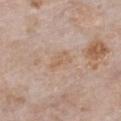Part of a total-body skin-imaging series; this lesion was reviewed on a skin check and was not flagged for biopsy. Located on the chest. A female patient about 85 years old or older. Longest diameter approximately 3 mm. Automated tile analysis of the lesion measured an average lesion color of about L≈61 a*≈17 b*≈30 (CIELAB), roughly 6 lightness units darker than nearby skin, and a normalized lesion–skin contrast near 5. It also reported a border-irregularity index near 3/10, a within-lesion color-variation index near 2/10, and a peripheral color-asymmetry measure near 0.5. It also reported a nevus-likeness score of about 0/100 and lesion-presence confidence of about 100/100. A lesion tile, about 15 mm wide, cut from a 3D total-body photograph. Captured under white-light illumination.